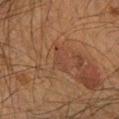Imaged during a routine full-body skin examination; the lesion was not biopsied and no histopathology is available. From the left forearm. A male subject roughly 50 years of age. An algorithmic analysis of the crop reported an average lesion color of about L≈33 a*≈18 b*≈25 (CIELAB) and a lesion–skin lightness drop of about 4. This is a cross-polarized tile. The lesion's longest dimension is about 3 mm. A lesion tile, about 15 mm wide, cut from a 3D total-body photograph.